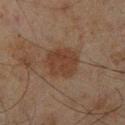Clinical impression: Recorded during total-body skin imaging; not selected for excision or biopsy. Background: A lesion tile, about 15 mm wide, cut from a 3D total-body photograph. Located on the leg. A male subject aged 43–47. The lesion's longest dimension is about 4.5 mm. This is a cross-polarized tile.